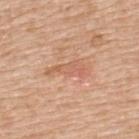  biopsy_status: not biopsied; imaged during a skin examination
  site: upper back
  lighting: white-light
  image:
    source: total-body photography crop
    field_of_view_mm: 15
  patient:
    sex: female
    age_approx: 55
  lesion_size:
    long_diameter_mm_approx: 5.0